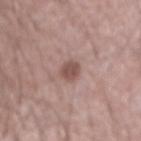Impression:
Recorded during total-body skin imaging; not selected for excision or biopsy.
Image and clinical context:
The lesion's longest dimension is about 2.5 mm. Imaged with white-light lighting. From the left forearm. Automated image analysis of the tile measured peripheral color asymmetry of about 0.5. A roughly 15 mm field-of-view crop from a total-body skin photograph. A male subject about 40 years old.